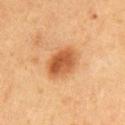The lesion was photographed on a routine skin check and not biopsied; there is no pathology result.
Cropped from a whole-body photographic skin survey; the tile spans about 15 mm.
The total-body-photography lesion software estimated an area of roughly 10 mm², a shape eccentricity near 0.7, and a shape-asymmetry score of about 0.1 (0 = symmetric). The analysis additionally found roughly 12 lightness units darker than nearby skin.
From the front of the torso.
The subject is a male approximately 55 years of age.